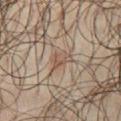{
  "biopsy_status": "not biopsied; imaged during a skin examination",
  "automated_metrics": {
    "cielab_L": 50,
    "cielab_a": 14,
    "cielab_b": 25,
    "vs_skin_darker_L": 7.0,
    "vs_skin_contrast_norm": 5.5,
    "border_irregularity_0_10": 3.5,
    "color_variation_0_10": 3.5,
    "peripheral_color_asymmetry": 1.0
  },
  "lesion_size": {
    "long_diameter_mm_approx": 3.5
  },
  "image": {
    "source": "total-body photography crop",
    "field_of_view_mm": 15
  },
  "site": "chest",
  "patient": {
    "sex": "male",
    "age_approx": 65
  },
  "lighting": "cross-polarized"
}